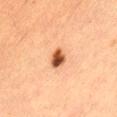Recorded during total-body skin imaging; not selected for excision or biopsy. From the lower back. A close-up tile cropped from a whole-body skin photograph, about 15 mm across. A female patient aged 53 to 57.